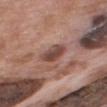– workup: imaged on a skin check; not biopsied
– imaging modality: total-body-photography crop, ~15 mm field of view
– subject: male, about 70 years old
– site: the upper back
– diameter: ≈3 mm
– automated metrics: a lesion area of about 6.5 mm² and an outline eccentricity of about 0.3 (0 = round, 1 = elongated); a border-irregularity index near 2/10, a color-variation rating of about 4.5/10, and peripheral color asymmetry of about 1.5; a lesion-detection confidence of about 100/100
– tile lighting: white-light